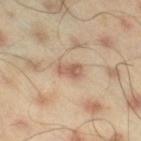The lesion was tiled from a total-body skin photograph and was not biopsied. The lesion is located on the right thigh. A male subject aged 43 to 47. A region of skin cropped from a whole-body photographic capture, roughly 15 mm wide.